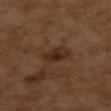Acquisition and patient details: The tile uses cross-polarized illumination. Approximately 3.5 mm at its widest. A close-up tile cropped from a whole-body skin photograph, about 15 mm across. The subject is a female about 55 years old. On the upper back.